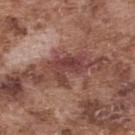Notes:
• workup — total-body-photography surveillance lesion; no biopsy
• image — total-body-photography crop, ~15 mm field of view
• anatomic site — the upper back
• patient — male, approximately 75 years of age